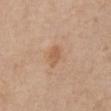Q: Was this lesion biopsied?
A: catalogued during a skin exam; not biopsied
Q: Where on the body is the lesion?
A: the chest
Q: How large is the lesion?
A: about 2.5 mm
Q: What is the imaging modality?
A: ~15 mm tile from a whole-body skin photo
Q: Automated lesion metrics?
A: an area of roughly 3.5 mm², a shape eccentricity near 0.8, and a symmetry-axis asymmetry near 0.25; lesion-presence confidence of about 100/100
Q: Who is the patient?
A: male, aged approximately 75
Q: What lighting was used for the tile?
A: white-light